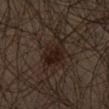| feature | finding |
|---|---|
| notes | total-body-photography surveillance lesion; no biopsy |
| body site | the front of the torso |
| size | ~5 mm (longest diameter) |
| image source | ~15 mm tile from a whole-body skin photo |
| patient | male, aged approximately 30 |
| lighting | cross-polarized illumination |